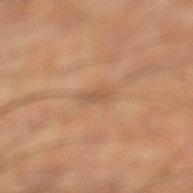Q: Is there a histopathology result?
A: catalogued during a skin exam; not biopsied
Q: What did automated image analysis measure?
A: a normalized lesion–skin contrast near 5; a border-irregularity rating of about 4.5/10, internal color variation of about 0 on a 0–10 scale, and radial color variation of about 0
Q: Where on the body is the lesion?
A: the left lower leg
Q: How large is the lesion?
A: ~3 mm (longest diameter)
Q: What is the imaging modality?
A: ~15 mm tile from a whole-body skin photo
Q: How was the tile lit?
A: cross-polarized
Q: What are the patient's age and sex?
A: male, in their 60s